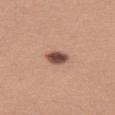* notes · imaged on a skin check; not biopsied
* body site · the left thigh
* acquisition · ~15 mm crop, total-body skin-cancer survey
* patient · female, aged approximately 45